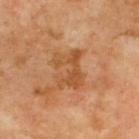{"biopsy_status": "not biopsied; imaged during a skin examination", "image": {"source": "total-body photography crop", "field_of_view_mm": 15}, "site": "back", "lighting": "cross-polarized", "lesion_size": {"long_diameter_mm_approx": 4.5}, "patient": {"sex": "male", "age_approx": 70}}